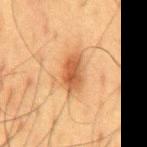| field | value |
|---|---|
| follow-up | catalogued during a skin exam; not biopsied |
| image-analysis metrics | an average lesion color of about L≈46 a*≈21 b*≈33 (CIELAB), a lesion–skin lightness drop of about 11, and a normalized lesion–skin contrast near 8.5; a border-irregularity index near 2/10 and a within-lesion color-variation index near 4/10; an automated nevus-likeness rating near 95 out of 100 and a lesion-detection confidence of about 100/100 |
| body site | the back |
| lighting | cross-polarized |
| image | ~15 mm crop, total-body skin-cancer survey |
| lesion diameter | ≈4.5 mm |
| patient | male, approximately 60 years of age |